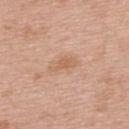Impression:
The lesion was tiled from a total-body skin photograph and was not biopsied.
Clinical summary:
On the upper back. Automated tile analysis of the lesion measured a footprint of about 3.5 mm² and an outline eccentricity of about 0.9 (0 = round, 1 = elongated). The analysis additionally found a mean CIELAB color near L≈61 a*≈21 b*≈33, about 7 CIELAB-L* units darker than the surrounding skin, and a normalized lesion–skin contrast near 6. The analysis additionally found a border-irregularity rating of about 5/10. And it measured a classifier nevus-likeness of about 0/100 and a lesion-detection confidence of about 100/100. The lesion's longest dimension is about 3 mm. A male patient, aged 63 to 67. This is a white-light tile. A region of skin cropped from a whole-body photographic capture, roughly 15 mm wide.